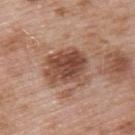Part of a total-body skin-imaging series; this lesion was reviewed on a skin check and was not flagged for biopsy. A region of skin cropped from a whole-body photographic capture, roughly 15 mm wide. A male subject aged 53–57. Located on the upper back.